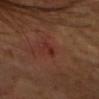The recorded lesion diameter is about 2.5 mm. From the head or neck. The patient is a female aged approximately 65. Cropped from a total-body skin-imaging series; the visible field is about 15 mm. The biopsy diagnosis was a squamous cell carcinoma in situ.